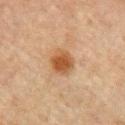| field | value |
|---|---|
| workup | catalogued during a skin exam; not biopsied |
| automated lesion analysis | border irregularity of about 1.5 on a 0–10 scale, internal color variation of about 3 on a 0–10 scale, and radial color variation of about 0.5; an automated nevus-likeness rating near 95 out of 100 and a lesion-detection confidence of about 100/100 |
| acquisition | ~15 mm tile from a whole-body skin photo |
| location | the upper back |
| diameter | ~3.5 mm (longest diameter) |
| subject | male, in their mid- to late 60s |